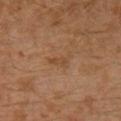Imaged during a routine full-body skin examination; the lesion was not biopsied and no histopathology is available.